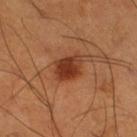size: ~3 mm (longest diameter); patient: male, aged around 55; anatomic site: the right thigh; image: 15 mm crop, total-body photography.